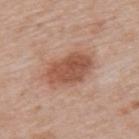Q: Was this lesion biopsied?
A: no biopsy performed (imaged during a skin exam)